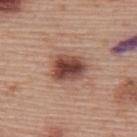Captured under white-light illumination. A female subject, aged approximately 60. Located on the upper back. A region of skin cropped from a whole-body photographic capture, roughly 15 mm wide. The lesion-visualizer software estimated a footprint of about 11 mm², an outline eccentricity of about 0.7 (0 = round, 1 = elongated), and a symmetry-axis asymmetry near 0.15. The analysis additionally found a lesion color around L≈46 a*≈22 b*≈26 in CIELAB, about 15 CIELAB-L* units darker than the surrounding skin, and a normalized lesion–skin contrast near 11. The software also gave a classifier nevus-likeness of about 95/100. Histopathologically confirmed as a dysplastic (Clark) nevus.